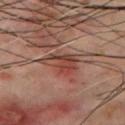Findings:
* follow-up — catalogued during a skin exam; not biopsied
* anatomic site — the chest
* subject — male, about 70 years old
* image source — ~15 mm tile from a whole-body skin photo
* tile lighting — cross-polarized illumination
* diameter — about 4.5 mm